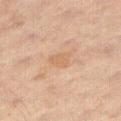Clinical impression: Captured during whole-body skin photography for melanoma surveillance; the lesion was not biopsied. Clinical summary: On the right thigh. This is a cross-polarized tile. The total-body-photography lesion software estimated a mean CIELAB color near L≈60 a*≈18 b*≈33, roughly 6 lightness units darker than nearby skin, and a normalized border contrast of about 5. And it measured border irregularity of about 2 on a 0–10 scale and a color-variation rating of about 1.5/10. The patient is a male aged 58–62. The lesion's longest dimension is about 2.5 mm. Cropped from a total-body skin-imaging series; the visible field is about 15 mm.